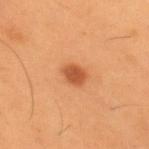The lesion was photographed on a routine skin check and not biopsied; there is no pathology result. Longest diameter approximately 2.5 mm. A 15 mm close-up tile from a total-body photography series done for melanoma screening. Automated tile analysis of the lesion measured a footprint of about 4.5 mm². The analysis additionally found an average lesion color of about L≈53 a*≈29 b*≈41 (CIELAB), a lesion–skin lightness drop of about 12, and a lesion-to-skin contrast of about 8 (normalized; higher = more distinct). And it measured lesion-presence confidence of about 100/100. A male patient, aged 53 to 57. The lesion is on the upper back.